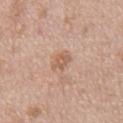{
  "biopsy_status": "not biopsied; imaged during a skin examination",
  "patient": {
    "sex": "male",
    "age_approx": 55
  },
  "site": "front of the torso",
  "automated_metrics": {
    "area_mm2_approx": 4.5,
    "eccentricity": 0.65,
    "shape_asymmetry": 0.25
  },
  "lesion_size": {
    "long_diameter_mm_approx": 3.0
  },
  "lighting": "white-light",
  "image": {
    "source": "total-body photography crop",
    "field_of_view_mm": 15
  }
}